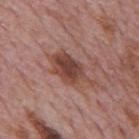The lesion was photographed on a routine skin check and not biopsied; there is no pathology result. The lesion's longest dimension is about 6 mm. The lesion is on the mid back. Automated tile analysis of the lesion measured a lesion color around L≈44 a*≈22 b*≈25 in CIELAB, about 11 CIELAB-L* units darker than the surrounding skin, and a normalized lesion–skin contrast near 9. A 15 mm crop from a total-body photograph taken for skin-cancer surveillance. A male subject, aged approximately 75. This is a white-light tile.